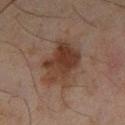No biopsy was performed on this lesion — it was imaged during a full skin examination and was not determined to be concerning. A male subject, aged around 45. A 15 mm crop from a total-body photograph taken for skin-cancer surveillance. The lesion is on the left lower leg. The tile uses cross-polarized illumination.